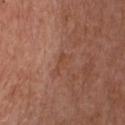| feature | finding |
|---|---|
| follow-up | catalogued during a skin exam; not biopsied |
| image-analysis metrics | a border-irregularity index near 5.5/10, a within-lesion color-variation index near 0/10, and radial color variation of about 0; a nevus-likeness score of about 0/100 and lesion-presence confidence of about 100/100 |
| image | ~15 mm tile from a whole-body skin photo |
| diameter | about 3 mm |
| patient | female, aged 73 to 77 |
| body site | the chest |
| tile lighting | white-light |